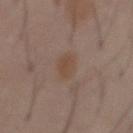Impression: The lesion was photographed on a routine skin check and not biopsied; there is no pathology result. Image and clinical context: From the front of the torso. Automated tile analysis of the lesion measured a border-irregularity rating of about 2/10, a color-variation rating of about 2/10, and radial color variation of about 0.5. And it measured an automated nevus-likeness rating near 10 out of 100 and a lesion-detection confidence of about 100/100. This image is a 15 mm lesion crop taken from a total-body photograph. A male patient, in their 60s.